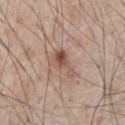biopsy status = total-body-photography surveillance lesion; no biopsy | imaging modality = ~15 mm crop, total-body skin-cancer survey | location = the chest | subject = male, about 65 years old.